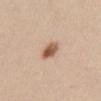Recorded during total-body skin imaging; not selected for excision or biopsy. Captured under white-light illumination. A 15 mm close-up tile from a total-body photography series done for melanoma screening. Approximately 3 mm at its widest. The subject is a female aged 23 to 27. From the abdomen.